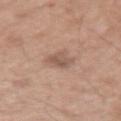The lesion was photographed on a routine skin check and not biopsied; there is no pathology result. From the arm. The total-body-photography lesion software estimated a mean CIELAB color near L≈55 a*≈19 b*≈26, about 9 CIELAB-L* units darker than the surrounding skin, and a normalized lesion–skin contrast near 6.5. The software also gave border irregularity of about 2.5 on a 0–10 scale and a within-lesion color-variation index near 3/10. A lesion tile, about 15 mm wide, cut from a 3D total-body photograph. The patient is a male roughly 50 years of age. Measured at roughly 2.5 mm in maximum diameter. This is a white-light tile.